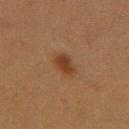The lesion was photographed on a routine skin check and not biopsied; there is no pathology result.
The lesion is located on the left thigh.
An algorithmic analysis of the crop reported border irregularity of about 2 on a 0–10 scale, internal color variation of about 2 on a 0–10 scale, and a peripheral color-asymmetry measure near 0.5. And it measured a nevus-likeness score of about 100/100.
This image is a 15 mm lesion crop taken from a total-body photograph.
The tile uses cross-polarized illumination.
Longest diameter approximately 3 mm.
The patient is a female aged 38–42.